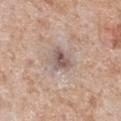The lesion was photographed on a routine skin check and not biopsied; there is no pathology result. The tile uses white-light illumination. Cropped from a whole-body photographic skin survey; the tile spans about 15 mm. A male subject, in their mid-60s. From the chest. The lesion's longest dimension is about 2.5 mm.